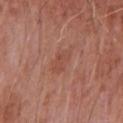Q: Was this lesion biopsied?
A: imaged on a skin check; not biopsied
Q: Who is the patient?
A: male, aged around 65
Q: Illumination type?
A: white-light illumination
Q: What did automated image analysis measure?
A: an area of roughly 5 mm², an outline eccentricity of about 0.75 (0 = round, 1 = elongated), and a symmetry-axis asymmetry near 0.3; a classifier nevus-likeness of about 0/100 and a detector confidence of about 100 out of 100 that the crop contains a lesion
Q: What is the anatomic site?
A: the chest
Q: What kind of image is this?
A: ~15 mm tile from a whole-body skin photo